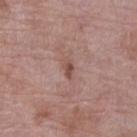  biopsy_status: not biopsied; imaged during a skin examination
  lesion_size:
    long_diameter_mm_approx: 2.5
  image:
    source: total-body photography crop
    field_of_view_mm: 15
  site: leg
  lighting: white-light
  patient:
    sex: female
    age_approx: 70